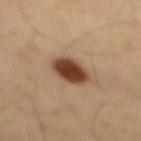• biopsy status · imaged on a skin check; not biopsied
• lesion size · ≈4 mm
• subject · male, aged 38–42
• tile lighting · cross-polarized illumination
• body site · the back
• image · 15 mm crop, total-body photography
• automated metrics · an average lesion color of about L≈42 a*≈20 b*≈31 (CIELAB), roughly 18 lightness units darker than nearby skin, and a normalized lesion–skin contrast near 13.5